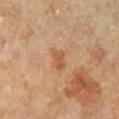{"biopsy_status": "not biopsied; imaged during a skin examination", "patient": {"sex": "female", "age_approx": 60}, "automated_metrics": {"area_mm2_approx": 3.5, "eccentricity": 0.8, "shape_asymmetry": 0.35}, "lesion_size": {"long_diameter_mm_approx": 2.5}, "image": {"source": "total-body photography crop", "field_of_view_mm": 15}, "site": "left lower leg", "lighting": "cross-polarized"}